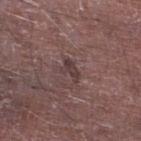follow-up: no biopsy performed (imaged during a skin exam); site: the right lower leg; subject: male, approximately 65 years of age; acquisition: ~15 mm crop, total-body skin-cancer survey.